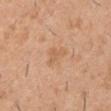Q: Was a biopsy performed?
A: no biopsy performed (imaged during a skin exam)
Q: Who is the patient?
A: male, in their 40s
Q: What kind of image is this?
A: ~15 mm tile from a whole-body skin photo
Q: How was the tile lit?
A: white-light
Q: What did automated image analysis measure?
A: an average lesion color of about L≈61 a*≈21 b*≈35 (CIELAB), about 7 CIELAB-L* units darker than the surrounding skin, and a normalized border contrast of about 4.5; a border-irregularity index near 6/10 and a within-lesion color-variation index near 0/10; an automated nevus-likeness rating near 0 out of 100 and lesion-presence confidence of about 100/100
Q: Where on the body is the lesion?
A: the arm